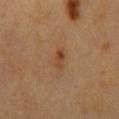Case summary:
– biopsy status: no biopsy performed (imaged during a skin exam)
– image source: ~15 mm crop, total-body skin-cancer survey
– size: about 3 mm
– site: the mid back
– lighting: cross-polarized illumination
– subject: male, aged 58–62